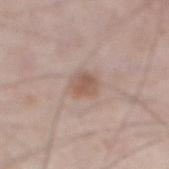Assessment:
No biopsy was performed on this lesion — it was imaged during a full skin examination and was not determined to be concerning.